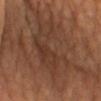notes=no biopsy performed (imaged during a skin exam) | subject=female, aged approximately 70 | diameter=about 17.5 mm | body site=the chest | image-analysis metrics=a lesion area of about 170 mm² and two-axis asymmetry of about 0.35; about 8 CIELAB-L* units darker than the surrounding skin; a classifier nevus-likeness of about 0/100 | imaging modality=~15 mm tile from a whole-body skin photo.